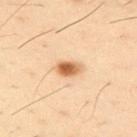Part of a total-body skin-imaging series; this lesion was reviewed on a skin check and was not flagged for biopsy. Measured at roughly 3 mm in maximum diameter. This is a cross-polarized tile. The lesion is located on the right upper arm. The patient is a male aged around 40. A region of skin cropped from a whole-body photographic capture, roughly 15 mm wide.